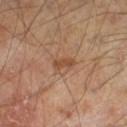Assessment:
Imaged during a routine full-body skin examination; the lesion was not biopsied and no histopathology is available.
Context:
The recorded lesion diameter is about 3 mm. The lesion is on the left lower leg. A male patient, about 65 years old. Cropped from a whole-body photographic skin survey; the tile spans about 15 mm.